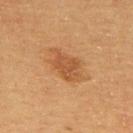The lesion was photographed on a routine skin check and not biopsied; there is no pathology result. From the back. The total-body-photography lesion software estimated a mean CIELAB color near L≈46 a*≈22 b*≈35 and a lesion–skin lightness drop of about 8. A male patient, roughly 60 years of age. Imaged with cross-polarized lighting. A region of skin cropped from a whole-body photographic capture, roughly 15 mm wide. Measured at roughly 4.5 mm in maximum diameter.